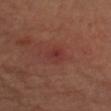biopsy_status: not biopsied; imaged during a skin examination
patient:
  sex: female
  age_approx: 45
automated_metrics:
  cielab_L: 33
  cielab_a: 26
  cielab_b: 23
  vs_skin_darker_L: 5.0
  vs_skin_contrast_norm: 5.0
  border_irregularity_0_10: 2.0
  color_variation_0_10: 4.5
  peripheral_color_asymmetry: 1.5
lesion_size:
  long_diameter_mm_approx: 2.5
image:
  source: total-body photography crop
  field_of_view_mm: 15
site: left upper arm
lighting: cross-polarized